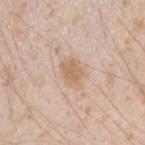Assessment: Recorded during total-body skin imaging; not selected for excision or biopsy. Image and clinical context: A male patient aged around 25. Cropped from a whole-body photographic skin survey; the tile spans about 15 mm. Automated image analysis of the tile measured a footprint of about 6 mm², an outline eccentricity of about 0.65 (0 = round, 1 = elongated), and two-axis asymmetry of about 0.2. The analysis additionally found a nevus-likeness score of about 15/100 and a lesion-detection confidence of about 100/100. Longest diameter approximately 3 mm. The lesion is on the right forearm. Imaged with white-light lighting.